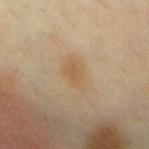Clinical impression: Recorded during total-body skin imaging; not selected for excision or biopsy. Acquisition and patient details: The tile uses cross-polarized illumination. A lesion tile, about 15 mm wide, cut from a 3D total-body photograph. Located on the chest. Measured at roughly 4 mm in maximum diameter. A female patient, aged around 40.